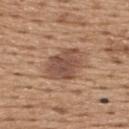Captured during whole-body skin photography for melanoma surveillance; the lesion was not biopsied. A 15 mm crop from a total-body photograph taken for skin-cancer surveillance. A male patient aged around 65. Automated image analysis of the tile measured a lesion area of about 13 mm² and a shape eccentricity near 0.7. It also reported a lesion color around L≈50 a*≈19 b*≈28 in CIELAB and a lesion-to-skin contrast of about 8 (normalized; higher = more distinct). The software also gave a border-irregularity rating of about 2/10 and a peripheral color-asymmetry measure near 1.5. And it measured a nevus-likeness score of about 65/100. Imaged with white-light lighting. The lesion is on the upper back.